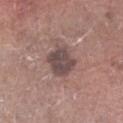notes: no biopsy performed (imaged during a skin exam) | lighting: white-light illumination | patient: male, about 60 years old | imaging modality: total-body-photography crop, ~15 mm field of view | anatomic site: the arm | automated lesion analysis: a shape eccentricity near 0.45 and a symmetry-axis asymmetry near 0.1; a mean CIELAB color near L≈46 a*≈16 b*≈17 and roughly 11 lightness units darker than nearby skin; a border-irregularity rating of about 1.5/10; a detector confidence of about 100 out of 100 that the crop contains a lesion.